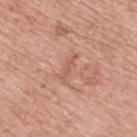  biopsy_status: not biopsied; imaged during a skin examination
  lesion_size:
    long_diameter_mm_approx: 3.5
  image:
    source: total-body photography crop
    field_of_view_mm: 15
  lighting: white-light
  patient:
    sex: male
    age_approx: 75
  site: upper back